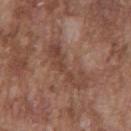Captured during whole-body skin photography for melanoma surveillance; the lesion was not biopsied. This image is a 15 mm lesion crop taken from a total-body photograph. Automated tile analysis of the lesion measured a lesion color around L≈44 a*≈20 b*≈26 in CIELAB, roughly 7 lightness units darker than nearby skin, and a normalized lesion–skin contrast near 6. Measured at roughly 6.5 mm in maximum diameter. Imaged with white-light lighting. A male subject, approximately 75 years of age. On the chest.